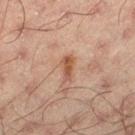No biopsy was performed on this lesion — it was imaged during a full skin examination and was not determined to be concerning. A male subject, approximately 50 years of age. A close-up tile cropped from a whole-body skin photograph, about 15 mm across. About 3 mm across. Automated tile analysis of the lesion measured an eccentricity of roughly 0.85 and a shape-asymmetry score of about 0.3 (0 = symmetric). It also reported an average lesion color of about L≈40 a*≈18 b*≈26 (CIELAB), roughly 8 lightness units darker than nearby skin, and a normalized lesion–skin contrast near 7.5. On the left thigh. The tile uses cross-polarized illumination.